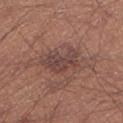This lesion was catalogued during total-body skin photography and was not selected for biopsy. A close-up tile cropped from a whole-body skin photograph, about 15 mm across. About 5 mm across. A male patient, approximately 45 years of age. The lesion is located on the left lower leg. Automated tile analysis of the lesion measured border irregularity of about 4 on a 0–10 scale, internal color variation of about 3.5 on a 0–10 scale, and peripheral color asymmetry of about 1.5. It also reported an automated nevus-likeness rating near 5 out of 100 and a detector confidence of about 100 out of 100 that the crop contains a lesion.